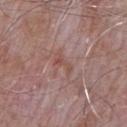<tbp_lesion>
  <biopsy_status>not biopsied; imaged during a skin examination</biopsy_status>
  <lesion_size>
    <long_diameter_mm_approx>3.0</long_diameter_mm_approx>
  </lesion_size>
  <site>left upper arm</site>
  <patient>
    <sex>male</sex>
    <age_approx>65</age_approx>
  </patient>
  <lighting>white-light</lighting>
  <image>
    <source>total-body photography crop</source>
    <field_of_view_mm>15</field_of_view_mm>
  </image>
</tbp_lesion>